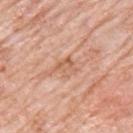<case>
<biopsy_status>not biopsied; imaged during a skin examination</biopsy_status>
<site>left upper arm</site>
<patient>
  <sex>male</sex>
  <age_approx>80</age_approx>
</patient>
<image>
  <source>total-body photography crop</source>
  <field_of_view_mm>15</field_of_view_mm>
</image>
<lighting>white-light</lighting>
<automated_metrics>
  <area_mm2_approx>4.5</area_mm2_approx>
</automated_metrics>
<lesion_size>
  <long_diameter_mm_approx>3.0</long_diameter_mm_approx>
</lesion_size>
</case>